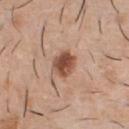Captured during whole-body skin photography for melanoma surveillance; the lesion was not biopsied. This is a white-light tile. An algorithmic analysis of the crop reported an area of roughly 7 mm², a shape eccentricity near 0.55, and a symmetry-axis asymmetry near 0.2. It also reported a lesion color around L≈50 a*≈22 b*≈30 in CIELAB, a lesion–skin lightness drop of about 15, and a normalized lesion–skin contrast near 10. And it measured a border-irregularity index near 1.5/10, internal color variation of about 4.5 on a 0–10 scale, and peripheral color asymmetry of about 1.5. The analysis additionally found an automated nevus-likeness rating near 100 out of 100. Located on the front of the torso. Approximately 3.5 mm at its widest. Cropped from a total-body skin-imaging series; the visible field is about 15 mm. A male subject approximately 40 years of age.